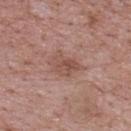A lesion tile, about 15 mm wide, cut from a 3D total-body photograph. This is a white-light tile. About 3.5 mm across. Automated tile analysis of the lesion measured a classifier nevus-likeness of about 0/100 and lesion-presence confidence of about 100/100. On the upper back. The subject is a male aged around 70.